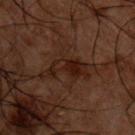• tile lighting — cross-polarized
• patient — male, about 50 years old
• imaging modality — ~15 mm tile from a whole-body skin photo
• body site — the chest